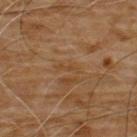illumination: cross-polarized | size: ~2.5 mm (longest diameter) | patient: male, in their 60s | image source: 15 mm crop, total-body photography | body site: the chest | automated lesion analysis: a footprint of about 2.5 mm², an eccentricity of roughly 0.95, and a shape-asymmetry score of about 0.35 (0 = symmetric); border irregularity of about 4.5 on a 0–10 scale, internal color variation of about 0 on a 0–10 scale, and peripheral color asymmetry of about 0.